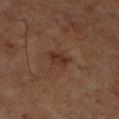Assessment:
This lesion was catalogued during total-body skin photography and was not selected for biopsy.
Context:
The patient is a male aged approximately 65. A close-up tile cropped from a whole-body skin photograph, about 15 mm across. Located on the left lower leg. About 2.5 mm across.